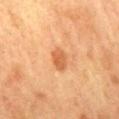Case summary:
- notes · total-body-photography surveillance lesion; no biopsy
- body site · the mid back
- patient · female, roughly 60 years of age
- lighting · cross-polarized
- acquisition · ~15 mm crop, total-body skin-cancer survey
- lesion diameter · about 3 mm
- automated metrics · about 10 CIELAB-L* units darker than the surrounding skin and a lesion-to-skin contrast of about 7 (normalized; higher = more distinct); a border-irregularity index near 1.5/10, a within-lesion color-variation index near 1.5/10, and a peripheral color-asymmetry measure near 0.5; an automated nevus-likeness rating near 90 out of 100 and a detector confidence of about 100 out of 100 that the crop contains a lesion